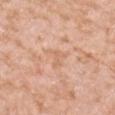biopsy_status: not biopsied; imaged during a skin examination
site: left forearm
patient:
  sex: female
  age_approx: 40
image:
  source: total-body photography crop
  field_of_view_mm: 15